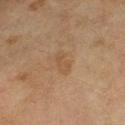The lesion was photographed on a routine skin check and not biopsied; there is no pathology result.
A 15 mm close-up tile from a total-body photography series done for melanoma screening.
On the right lower leg.
The subject is a female aged 58–62.